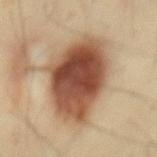Recorded during total-body skin imaging; not selected for excision or biopsy.
The lesion-visualizer software estimated an area of roughly 39 mm² and two-axis asymmetry of about 0.15. It also reported a mean CIELAB color near L≈44 a*≈19 b*≈28, roughly 17 lightness units darker than nearby skin, and a normalized border contrast of about 12.5. The software also gave a border-irregularity index near 2/10 and a peripheral color-asymmetry measure near 2.
This image is a 15 mm lesion crop taken from a total-body photograph.
A male patient, about 50 years old.
The lesion is located on the mid back.
The recorded lesion diameter is about 8.5 mm.
This is a cross-polarized tile.